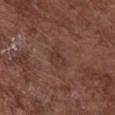Q: Where on the body is the lesion?
A: the chest
Q: How large is the lesion?
A: ≈3 mm
Q: Patient demographics?
A: female, about 80 years old
Q: What kind of image is this?
A: total-body-photography crop, ~15 mm field of view
Q: How was the tile lit?
A: white-light illumination